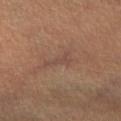follow-up = catalogued during a skin exam; not biopsied | lesion diameter = ~4 mm (longest diameter) | image-analysis metrics = a lesion area of about 4.5 mm², a shape eccentricity near 0.95, and two-axis asymmetry of about 0.55; a normalized border contrast of about 5; a border-irregularity rating of about 6.5/10 and peripheral color asymmetry of about 0.5; a classifier nevus-likeness of about 0/100 and a lesion-detection confidence of about 55/100 | tile lighting = cross-polarized illumination | imaging modality = 15 mm crop, total-body photography | site = the leg | patient = female, roughly 30 years of age.